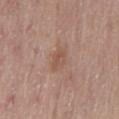| key | value |
|---|---|
| biopsy status | no biopsy performed (imaged during a skin exam) |
| lesion diameter | ≈3 mm |
| acquisition | ~15 mm tile from a whole-body skin photo |
| subject | male, aged 53 to 57 |
| anatomic site | the lower back |
| tile lighting | white-light illumination |